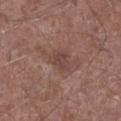  biopsy_status: not biopsied; imaged during a skin examination
  lighting: white-light
  lesion_size:
    long_diameter_mm_approx: 2.5
  image:
    source: total-body photography crop
    field_of_view_mm: 15
  site: left lower leg
  patient:
    sex: male
    age_approx: 45